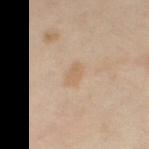<lesion>
  <biopsy_status>not biopsied; imaged during a skin examination</biopsy_status>
  <image>
    <source>total-body photography crop</source>
    <field_of_view_mm>15</field_of_view_mm>
  </image>
  <lighting>cross-polarized</lighting>
  <site>right thigh</site>
  <lesion_size>
    <long_diameter_mm_approx>2.5</long_diameter_mm_approx>
  </lesion_size>
  <patient>
    <sex>female</sex>
    <age_approx>50</age_approx>
  </patient>
</lesion>